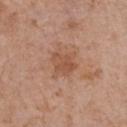follow-up = imaged on a skin check; not biopsied | lesion size = ≈3 mm | imaging modality = ~15 mm tile from a whole-body skin photo | body site = the front of the torso | patient = female, aged 63–67.